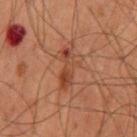About 5 mm across. The tile uses cross-polarized illumination. A lesion tile, about 15 mm wide, cut from a 3D total-body photograph. The lesion is on the back. The patient is a male aged 58 to 62.